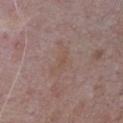{"lesion_size": {"long_diameter_mm_approx": 4.0}, "patient": {"sex": "male", "age_approx": 65}, "site": "chest", "automated_metrics": {"area_mm2_approx": 5.0, "shape_asymmetry": 0.6, "cielab_L": 51, "cielab_a": 17, "cielab_b": 23, "vs_skin_darker_L": 4.0, "vs_skin_contrast_norm": 4.5, "nevus_likeness_0_100": 0, "lesion_detection_confidence_0_100": 100}, "lighting": "white-light", "image": {"source": "total-body photography crop", "field_of_view_mm": 15}}